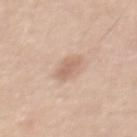Q: Is there a histopathology result?
A: no biopsy performed (imaged during a skin exam)
Q: Lesion location?
A: the front of the torso
Q: What lighting was used for the tile?
A: white-light illumination
Q: How was this image acquired?
A: ~15 mm tile from a whole-body skin photo
Q: Lesion size?
A: about 3 mm
Q: What are the patient's age and sex?
A: male, aged 48–52